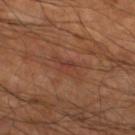Imaged during a routine full-body skin examination; the lesion was not biopsied and no histopathology is available.
A 15 mm crop from a total-body photograph taken for skin-cancer surveillance.
Imaged with cross-polarized lighting.
The lesion is on the left lower leg.
A male patient, about 60 years old.